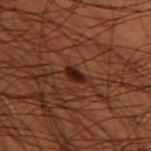workup = catalogued during a skin exam; not biopsied | subject = male, aged 48–52 | anatomic site = the left thigh | image source = total-body-photography crop, ~15 mm field of view.